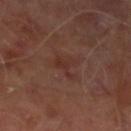image = 15 mm crop, total-body photography; body site = the leg; patient = male, in their mid- to late 60s; illumination = cross-polarized illumination.